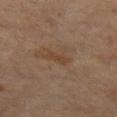Imaged during a routine full-body skin examination; the lesion was not biopsied and no histopathology is available. A male patient aged around 60. A lesion tile, about 15 mm wide, cut from a 3D total-body photograph. The total-body-photography lesion software estimated a border-irregularity index near 3/10 and a peripheral color-asymmetry measure near 0. The software also gave a nevus-likeness score of about 0/100 and a detector confidence of about 100 out of 100 that the crop contains a lesion. Imaged with cross-polarized lighting. The lesion is located on the left thigh.